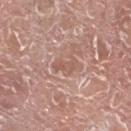Q: Was this lesion biopsied?
A: catalogued during a skin exam; not biopsied
Q: Who is the patient?
A: male, approximately 75 years of age
Q: What is the anatomic site?
A: the left lower leg
Q: Automated lesion metrics?
A: a mean CIELAB color near L≈56 a*≈22 b*≈26 and a lesion-to-skin contrast of about 5 (normalized; higher = more distinct); an automated nevus-likeness rating near 0 out of 100 and lesion-presence confidence of about 80/100
Q: What lighting was used for the tile?
A: white-light illumination
Q: How was this image acquired?
A: total-body-photography crop, ~15 mm field of view